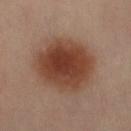Assessment: Captured during whole-body skin photography for melanoma surveillance; the lesion was not biopsied. Background: From the right thigh. A female subject aged approximately 40. This image is a 15 mm lesion crop taken from a total-body photograph. The tile uses cross-polarized illumination. The recorded lesion diameter is about 7 mm.